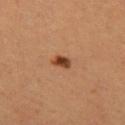follow-up = total-body-photography surveillance lesion; no biopsy | automated lesion analysis = a lesion color around L≈42 a*≈25 b*≈35 in CIELAB, a lesion–skin lightness drop of about 15, and a normalized lesion–skin contrast near 11; a border-irregularity rating of about 2.5/10, a within-lesion color-variation index near 3/10, and peripheral color asymmetry of about 1 | acquisition = ~15 mm tile from a whole-body skin photo | patient = female, about 25 years old | location = the left thigh | diameter = ~2.5 mm (longest diameter).